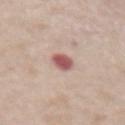notes: imaged on a skin check; not biopsied | image-analysis metrics: a lesion area of about 4.5 mm²; an average lesion color of about L≈56 a*≈26 b*≈22 (CIELAB), roughly 15 lightness units darker than nearby skin, and a normalized border contrast of about 10; a border-irregularity rating of about 2/10, a within-lesion color-variation index near 2.5/10, and radial color variation of about 1 | image: 15 mm crop, total-body photography | subject: female, about 75 years old | size: ~3 mm (longest diameter) | anatomic site: the abdomen | tile lighting: white-light illumination.